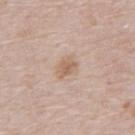* follow-up · catalogued during a skin exam; not biopsied
* lesion size · ≈3 mm
* image-analysis metrics · an area of roughly 4 mm², a shape eccentricity near 0.75, and a symmetry-axis asymmetry near 0.3; an average lesion color of about L≈61 a*≈17 b*≈29 (CIELAB) and a normalized border contrast of about 6.5; a border-irregularity index near 2.5/10, a within-lesion color-variation index near 3/10, and radial color variation of about 1
* patient · male, in their 80s
* lighting · white-light
* image · 15 mm crop, total-body photography
* site · the abdomen